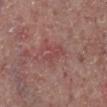<case>
  <biopsy_status>not biopsied; imaged during a skin examination</biopsy_status>
  <image>
    <source>total-body photography crop</source>
    <field_of_view_mm>15</field_of_view_mm>
  </image>
  <lighting>white-light</lighting>
  <patient>
    <sex>male</sex>
    <age_approx>65</age_approx>
  </patient>
  <lesion_size>
    <long_diameter_mm_approx>3.5</long_diameter_mm_approx>
  </lesion_size>
  <automated_metrics>
    <nevus_likeness_0_100>0</nevus_likeness_0_100>
    <lesion_detection_confidence_0_100>100</lesion_detection_confidence_0_100>
  </automated_metrics>
  <site>left lower leg</site>
</case>